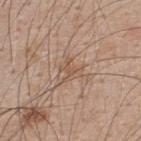Q: Was a biopsy performed?
A: total-body-photography surveillance lesion; no biopsy
Q: Where on the body is the lesion?
A: the upper back
Q: Who is the patient?
A: male, aged 48–52
Q: How was this image acquired?
A: 15 mm crop, total-body photography
Q: What is the lesion's diameter?
A: ~2.5 mm (longest diameter)
Q: How was the tile lit?
A: white-light illumination